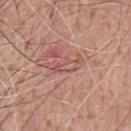{
  "biopsy_status": "not biopsied; imaged during a skin examination",
  "image": {
    "source": "total-body photography crop",
    "field_of_view_mm": 15
  },
  "lighting": "white-light",
  "patient": {
    "sex": "male",
    "age_approx": 60
  },
  "site": "chest"
}